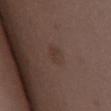A 15 mm close-up extracted from a 3D total-body photography capture.
A female patient aged approximately 35.
Located on the abdomen.